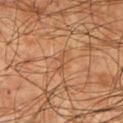Imaged during a routine full-body skin examination; the lesion was not biopsied and no histopathology is available. A 15 mm close-up tile from a total-body photography series done for melanoma screening. A male subject in their mid-70s. On the right upper arm.